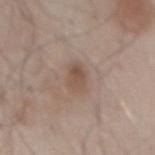Q: Was a biopsy performed?
A: no biopsy performed (imaged during a skin exam)
Q: What is the anatomic site?
A: the mid back
Q: Who is the patient?
A: male, in their mid-50s
Q: What is the imaging modality?
A: ~15 mm crop, total-body skin-cancer survey